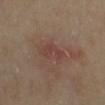The lesion was photographed on a routine skin check and not biopsied; there is no pathology result.
Imaged with cross-polarized lighting.
A female subject, about 70 years old.
Cropped from a total-body skin-imaging series; the visible field is about 15 mm.
From the leg.